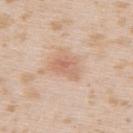| field | value |
|---|---|
| biopsy status | imaged on a skin check; not biopsied |
| subject | female, in their mid-20s |
| acquisition | ~15 mm crop, total-body skin-cancer survey |
| site | the upper back |
| lighting | white-light illumination |
| diameter | ≈3.5 mm |